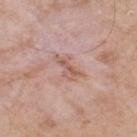  biopsy_status: not biopsied; imaged during a skin examination
  lighting: white-light
  patient:
    sex: male
    age_approx: 55
  image:
    source: total-body photography crop
    field_of_view_mm: 15
  site: upper back